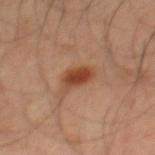Case summary:
- biopsy status · catalogued during a skin exam; not biopsied
- lesion diameter · ≈3 mm
- tile lighting · cross-polarized
- acquisition · 15 mm crop, total-body photography
- body site · the mid back
- patient · male, in their mid- to late 40s